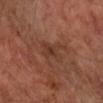Q: Was this lesion biopsied?
A: catalogued during a skin exam; not biopsied
Q: What kind of image is this?
A: ~15 mm crop, total-body skin-cancer survey
Q: How was the tile lit?
A: cross-polarized
Q: Where on the body is the lesion?
A: the right forearm
Q: What are the patient's age and sex?
A: male, aged approximately 65
Q: How large is the lesion?
A: ~3 mm (longest diameter)